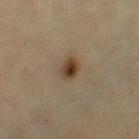<record>
<biopsy_status>not biopsied; imaged during a skin examination</biopsy_status>
<site>right thigh</site>
<patient>
  <sex>female</sex>
  <age_approx>65</age_approx>
</patient>
<image>
  <source>total-body photography crop</source>
  <field_of_view_mm>15</field_of_view_mm>
</image>
<lesion_size>
  <long_diameter_mm_approx>3.0</long_diameter_mm_approx>
</lesion_size>
<automated_metrics>
  <nevus_likeness_0_100>100</nevus_likeness_0_100>
  <lesion_detection_confidence_0_100>100</lesion_detection_confidence_0_100>
</automated_metrics>
</record>